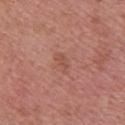Captured during whole-body skin photography for melanoma surveillance; the lesion was not biopsied.
Located on the upper back.
A female subject aged 48 to 52.
A 15 mm close-up tile from a total-body photography series done for melanoma screening.